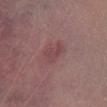Notes:
- biopsy status — imaged on a skin check; not biopsied
- acquisition — ~15 mm tile from a whole-body skin photo
- diameter — ~3 mm (longest diameter)
- site — the left lower leg
- patient — male, roughly 65 years of age
- lighting — cross-polarized
- automated lesion analysis — a normalized lesion–skin contrast near 5.5; a border-irregularity rating of about 5.5/10 and a peripheral color-asymmetry measure near 0.5